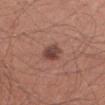notes=imaged on a skin check; not biopsied
imaging modality=~15 mm crop, total-body skin-cancer survey
lighting=white-light
site=the arm
TBP lesion metrics=an average lesion color of about L≈44 a*≈22 b*≈24 (CIELAB) and a lesion–skin lightness drop of about 11; an automated nevus-likeness rating near 75 out of 100 and a detector confidence of about 100 out of 100 that the crop contains a lesion
lesion size=about 3 mm
patient=male, approximately 30 years of age